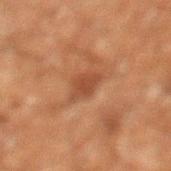  biopsy_status: not biopsied; imaged during a skin examination
  lighting: cross-polarized
  site: left lower leg
  patient:
    sex: male
    age_approx: 60
  automated_metrics:
    area_mm2_approx: 4.5
    eccentricity: 0.65
    shape_asymmetry: 0.3
    cielab_L: 36
    cielab_a: 20
    cielab_b: 28
    vs_skin_darker_L: 7.0
    vs_skin_contrast_norm: 6.5
    nevus_likeness_0_100: 0
    lesion_detection_confidence_0_100: 100
  image:
    source: total-body photography crop
    field_of_view_mm: 15
  lesion_size:
    long_diameter_mm_approx: 2.5